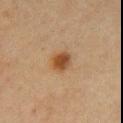Case summary:
- automated metrics: a mean CIELAB color near L≈41 a*≈18 b*≈32 and roughly 11 lightness units darker than nearby skin; a nevus-likeness score of about 100/100 and a detector confidence of about 100 out of 100 that the crop contains a lesion
- lighting: cross-polarized
- acquisition: total-body-photography crop, ~15 mm field of view
- patient: female, aged approximately 50
- size: ~2.5 mm (longest diameter)
- location: the chest